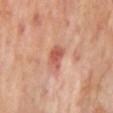* biopsy status · total-body-photography surveillance lesion; no biopsy
* lighting · cross-polarized
* acquisition · total-body-photography crop, ~15 mm field of view
* anatomic site · the mid back
* patient · male, aged approximately 65
* diameter · ≈2.5 mm
* automated lesion analysis · a shape eccentricity near 0.65; a mean CIELAB color near L≈53 a*≈29 b*≈29, about 11 CIELAB-L* units darker than the surrounding skin, and a lesion-to-skin contrast of about 7.5 (normalized; higher = more distinct); a border-irregularity index near 2.5/10, a within-lesion color-variation index near 2/10, and a peripheral color-asymmetry measure near 0.5; lesion-presence confidence of about 100/100